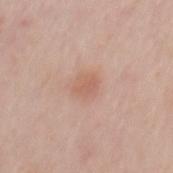Q: Was a biopsy performed?
A: imaged on a skin check; not biopsied
Q: How large is the lesion?
A: about 2.5 mm
Q: What are the patient's age and sex?
A: male, aged approximately 40
Q: Where on the body is the lesion?
A: the mid back
Q: What kind of image is this?
A: 15 mm crop, total-body photography
Q: What did automated image analysis measure?
A: an outline eccentricity of about 0.45 (0 = round, 1 = elongated) and two-axis asymmetry of about 0.2; about 7 CIELAB-L* units darker than the surrounding skin and a normalized border contrast of about 5; border irregularity of about 2 on a 0–10 scale, internal color variation of about 2 on a 0–10 scale, and radial color variation of about 0.5
Q: Illumination type?
A: white-light